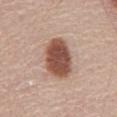<record>
  <biopsy_status>not biopsied; imaged during a skin examination</biopsy_status>
  <image>
    <source>total-body photography crop</source>
    <field_of_view_mm>15</field_of_view_mm>
  </image>
  <automated_metrics>
    <area_mm2_approx>16.0</area_mm2_approx>
    <eccentricity>0.75</eccentricity>
    <shape_asymmetry>0.15</shape_asymmetry>
    <cielab_L>51</cielab_L>
    <cielab_a>21</cielab_a>
    <cielab_b>27</cielab_b>
    <vs_skin_darker_L>17.0</vs_skin_darker_L>
    <color_variation_0_10>4.0</color_variation_0_10>
    <peripheral_color_asymmetry>1.0</peripheral_color_asymmetry>
  </automated_metrics>
  <patient>
    <sex>male</sex>
    <age_approx>60</age_approx>
  </patient>
  <site>abdomen</site>
</record>